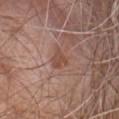Case summary:
- notes · imaged on a skin check; not biopsied
- image source · ~15 mm crop, total-body skin-cancer survey
- image-analysis metrics · a border-irregularity rating of about 3.5/10 and radial color variation of about 0.5; an automated nevus-likeness rating near 0 out of 100
- lighting · white-light
- lesion size · about 3 mm
- subject · male, roughly 60 years of age
- location · the mid back